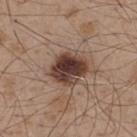Notes:
- workup: no biopsy performed (imaged during a skin exam)
- imaging modality: ~15 mm crop, total-body skin-cancer survey
- patient: male, aged 48 to 52
- lesion size: about 4.5 mm
- location: the upper back
- lighting: white-light